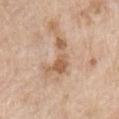The lesion was photographed on a routine skin check and not biopsied; there is no pathology result. A male patient, aged 58–62. This is a white-light tile. The lesion is on the chest. A roughly 15 mm field-of-view crop from a total-body skin photograph.